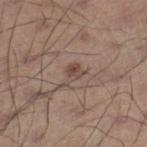Imaged during a routine full-body skin examination; the lesion was not biopsied and no histopathology is available.
A 15 mm crop from a total-body photograph taken for skin-cancer surveillance.
The lesion is on the right lower leg.
A male subject aged 53–57.